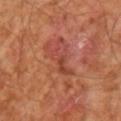automated_metrics:
  vs_skin_contrast_norm: 5.0
  peripheral_color_asymmetry: 1.5
lighting: cross-polarized
site: arm
image:
  source: total-body photography crop
  field_of_view_mm: 15
patient:
  sex: male
  age_approx: 65
lesion_size:
  long_diameter_mm_approx: 7.0